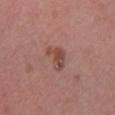The lesion was tiled from a total-body skin photograph and was not biopsied. On the left lower leg. A 15 mm close-up extracted from a 3D total-body photography capture. The patient is a male approximately 70 years of age.